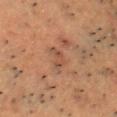* notes · no biopsy performed (imaged during a skin exam)
* subject · male, in their 60s
* image source · 15 mm crop, total-body photography
* lighting · cross-polarized illumination
* site · the head or neck
* lesion size · about 3 mm